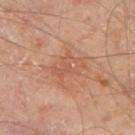Measured at roughly 4 mm in maximum diameter.
The total-body-photography lesion software estimated an eccentricity of roughly 0.7. The analysis additionally found a nevus-likeness score of about 0/100 and a detector confidence of about 100 out of 100 that the crop contains a lesion.
Located on the mid back.
A male subject in their mid-60s.
Cropped from a total-body skin-imaging series; the visible field is about 15 mm.